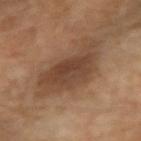notes = no biopsy performed (imaged during a skin exam) | patient = female, roughly 70 years of age | image = 15 mm crop, total-body photography | tile lighting = cross-polarized illumination | body site = the right forearm | automated lesion analysis = a footprint of about 24 mm²; an average lesion color of about L≈41 a*≈17 b*≈28 (CIELAB) and roughly 9 lightness units darker than nearby skin; a nevus-likeness score of about 25/100 and a detector confidence of about 100 out of 100 that the crop contains a lesion.